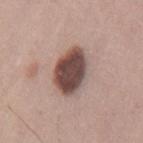Clinical impression: The lesion was photographed on a routine skin check and not biopsied; there is no pathology result. Image and clinical context: The lesion's longest dimension is about 5 mm. Imaged with white-light lighting. Located on the mid back. The total-body-photography lesion software estimated border irregularity of about 1.5 on a 0–10 scale and a within-lesion color-variation index near 5/10. The software also gave a detector confidence of about 100 out of 100 that the crop contains a lesion. The subject is a male in their mid- to late 40s. A 15 mm close-up extracted from a 3D total-body photography capture.